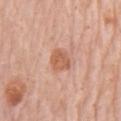Recorded during total-body skin imaging; not selected for excision or biopsy. Approximately 3 mm at its widest. A roughly 15 mm field-of-view crop from a total-body skin photograph. A male patient approximately 80 years of age. An algorithmic analysis of the crop reported a nevus-likeness score of about 25/100 and lesion-presence confidence of about 100/100. From the chest.